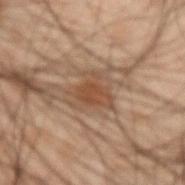The lesion was photographed on a routine skin check and not biopsied; there is no pathology result.
Captured under cross-polarized illumination.
A roughly 15 mm field-of-view crop from a total-body skin photograph.
Located on the left forearm.
A male patient, aged 48–52.
Longest diameter approximately 3.5 mm.
The total-body-photography lesion software estimated a footprint of about 7.5 mm², a shape eccentricity near 0.3, and two-axis asymmetry of about 0.5. And it measured a border-irregularity index near 6/10.